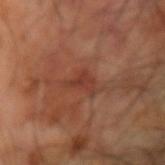<tbp_lesion>
  <biopsy_status>not biopsied; imaged during a skin examination</biopsy_status>
  <patient>
    <sex>male</sex>
    <age_approx>60</age_approx>
  </patient>
  <automated_metrics>
    <border_irregularity_0_10>6.0</border_irregularity_0_10>
    <color_variation_0_10>2.0</color_variation_0_10>
    <peripheral_color_asymmetry>0.5</peripheral_color_asymmetry>
    <nevus_likeness_0_100>5</nevus_likeness_0_100>
  </automated_metrics>
  <site>left arm</site>
  <image>
    <source>total-body photography crop</source>
    <field_of_view_mm>15</field_of_view_mm>
  </image>
</tbp_lesion>